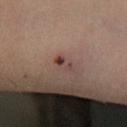Impression:
The lesion was photographed on a routine skin check and not biopsied; there is no pathology result.
Context:
Approximately 2.5 mm at its widest. A female patient, aged around 35. On the leg. A 15 mm close-up extracted from a 3D total-body photography capture. The tile uses cross-polarized illumination.